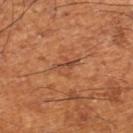The lesion was tiled from a total-body skin photograph and was not biopsied. Located on the right lower leg. The subject is a male aged approximately 65. This image is a 15 mm lesion crop taken from a total-body photograph. Captured under cross-polarized illumination. The lesion-visualizer software estimated an average lesion color of about L≈48 a*≈25 b*≈35 (CIELAB), about 7 CIELAB-L* units darker than the surrounding skin, and a normalized border contrast of about 5.5. Measured at roughly 3.5 mm in maximum diameter.